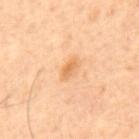Captured during whole-body skin photography for melanoma surveillance; the lesion was not biopsied. On the back. The lesion's longest dimension is about 2.5 mm. Cropped from a whole-body photographic skin survey; the tile spans about 15 mm. A male patient, approximately 60 years of age. This is a cross-polarized tile.